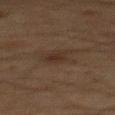Recorded during total-body skin imaging; not selected for excision or biopsy.
A male patient, approximately 65 years of age.
The lesion is on the mid back.
An algorithmic analysis of the crop reported a shape eccentricity near 0.7 and a shape-asymmetry score of about 0.35 (0 = symmetric). And it measured a border-irregularity rating of about 3.5/10, a within-lesion color-variation index near 1.5/10, and a peripheral color-asymmetry measure near 0.5. The analysis additionally found a nevus-likeness score of about 25/100 and a lesion-detection confidence of about 100/100.
Captured under cross-polarized illumination.
A 15 mm crop from a total-body photograph taken for skin-cancer surveillance.